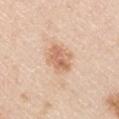follow-up — catalogued during a skin exam; not biopsied
imaging modality — ~15 mm crop, total-body skin-cancer survey
subject — male, roughly 45 years of age
size — ≈3.5 mm
anatomic site — the left upper arm
automated lesion analysis — an average lesion color of about L≈66 a*≈21 b*≈33 (CIELAB) and about 11 CIELAB-L* units darker than the surrounding skin; a border-irregularity index near 2/10, internal color variation of about 4.5 on a 0–10 scale, and radial color variation of about 1.5